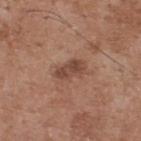Clinical impression:
No biopsy was performed on this lesion — it was imaged during a full skin examination and was not determined to be concerning.
Background:
A male patient, approximately 55 years of age. Imaged with white-light lighting. A region of skin cropped from a whole-body photographic capture, roughly 15 mm wide. The total-body-photography lesion software estimated a lesion area of about 6 mm², a shape eccentricity near 0.8, and a shape-asymmetry score of about 0.2 (0 = symmetric). And it measured a lesion color around L≈46 a*≈21 b*≈27 in CIELAB and about 9 CIELAB-L* units darker than the surrounding skin. The analysis additionally found a border-irregularity rating of about 2.5/10, internal color variation of about 3 on a 0–10 scale, and radial color variation of about 1. From the back. About 3.5 mm across.